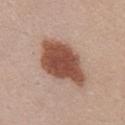patient: female, in their mid- to late 20s; lesion size: about 7.5 mm; location: the chest; acquisition: ~15 mm tile from a whole-body skin photo.